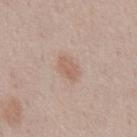Case summary:
– workup: total-body-photography surveillance lesion; no biopsy
– site: the abdomen
– patient: male, aged approximately 60
– image source: ~15 mm crop, total-body skin-cancer survey
– lesion size: about 3 mm